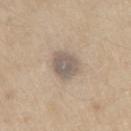  biopsy_status: not biopsied; imaged during a skin examination
  patient:
    sex: male
    age_approx: 70
  lighting: white-light
  lesion_size:
    long_diameter_mm_approx: 3.5
  image:
    source: total-body photography crop
    field_of_view_mm: 15
  site: left upper arm